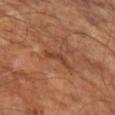Part of a total-body skin-imaging series; this lesion was reviewed on a skin check and was not flagged for biopsy. Approximately 3 mm at its widest. The total-body-photography lesion software estimated a lesion area of about 3 mm² and an eccentricity of roughly 0.9. The software also gave a lesion-to-skin contrast of about 6.5 (normalized; higher = more distinct). And it measured border irregularity of about 6 on a 0–10 scale and a within-lesion color-variation index near 0/10. A male subject, aged 63–67. A roughly 15 mm field-of-view crop from a total-body skin photograph. The lesion is located on the left upper arm.